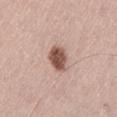<tbp_lesion>
<biopsy_status>not biopsied; imaged during a skin examination</biopsy_status>
<image>
  <source>total-body photography crop</source>
  <field_of_view_mm>15</field_of_view_mm>
</image>
<patient>
  <sex>male</sex>
  <age_approx>45</age_approx>
</patient>
<site>left thigh</site>
<lighting>white-light</lighting>
<lesion_size>
  <long_diameter_mm_approx>3.0</long_diameter_mm_approx>
</lesion_size>
<automated_metrics>
  <area_mm2_approx>7.0</area_mm2_approx>
  <eccentricity>0.65</eccentricity>
  <shape_asymmetry>0.15</shape_asymmetry>
  <cielab_L>53</cielab_L>
  <cielab_a>20</cielab_a>
  <cielab_b>25</cielab_b>
  <vs_skin_contrast_norm>11.0</vs_skin_contrast_norm>
  <nevus_likeness_0_100>95</nevus_likeness_0_100>
  <lesion_detection_confidence_0_100>100</lesion_detection_confidence_0_100>
</automated_metrics>
</tbp_lesion>